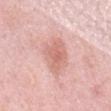Findings:
– workup · no biopsy performed (imaged during a skin exam)
– location · the abdomen
– image source · ~15 mm tile from a whole-body skin photo
– patient · male, aged approximately 55
– illumination · white-light illumination
– TBP lesion metrics · a lesion area of about 12 mm² and a symmetry-axis asymmetry near 0.25; an average lesion color of about L≈66 a*≈24 b*≈26 (CIELAB), a lesion–skin lightness drop of about 9, and a normalized border contrast of about 6; internal color variation of about 2.5 on a 0–10 scale and peripheral color asymmetry of about 0.5